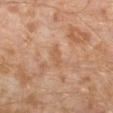A male patient aged approximately 30.
A 15 mm close-up extracted from a 3D total-body photography capture.
The lesion is on the left lower leg.